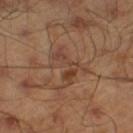Part of a total-body skin-imaging series; this lesion was reviewed on a skin check and was not flagged for biopsy.
From the left lower leg.
The patient is a male aged around 45.
Captured under cross-polarized illumination.
A 15 mm close-up tile from a total-body photography series done for melanoma screening.
Automated tile analysis of the lesion measured border irregularity of about 9 on a 0–10 scale, internal color variation of about 3 on a 0–10 scale, and radial color variation of about 0.
Approximately 4.5 mm at its widest.